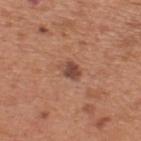biopsy status: total-body-photography surveillance lesion; no biopsy | lesion size: ≈2.5 mm | image-analysis metrics: a lesion color around L≈47 a*≈23 b*≈28 in CIELAB | illumination: white-light illumination | patient: male, in their mid- to late 60s | location: the upper back | acquisition: ~15 mm crop, total-body skin-cancer survey.